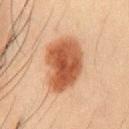workup = no biopsy performed (imaged during a skin exam)
subject = male, aged 58 to 62
anatomic site = the chest
imaging modality = ~15 mm tile from a whole-body skin photo